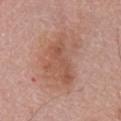No biopsy was performed on this lesion — it was imaged during a full skin examination and was not determined to be concerning.
Cropped from a whole-body photographic skin survey; the tile spans about 15 mm.
A male subject in their 80s.
The tile uses white-light illumination.
The lesion's longest dimension is about 8 mm.
The lesion is on the chest.
Automated tile analysis of the lesion measured a footprint of about 30 mm² and two-axis asymmetry of about 0.25. And it measured a mean CIELAB color near L≈56 a*≈21 b*≈29, about 8 CIELAB-L* units darker than the surrounding skin, and a normalized border contrast of about 6. It also reported lesion-presence confidence of about 100/100.